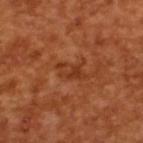Impression: Imaged during a routine full-body skin examination; the lesion was not biopsied and no histopathology is available. Background: An algorithmic analysis of the crop reported an outline eccentricity of about 0.85 (0 = round, 1 = elongated) and two-axis asymmetry of about 0.25. And it measured a lesion color around L≈36 a*≈27 b*≈36 in CIELAB. And it measured a border-irregularity index near 3.5/10. A male patient approximately 65 years of age. A region of skin cropped from a whole-body photographic capture, roughly 15 mm wide. The tile uses cross-polarized illumination.